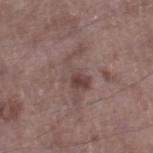Clinical impression: Captured during whole-body skin photography for melanoma surveillance; the lesion was not biopsied.